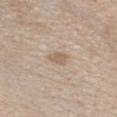{"biopsy_status": "not biopsied; imaged during a skin examination", "lighting": "white-light", "image": {"source": "total-body photography crop", "field_of_view_mm": 15}, "site": "front of the torso", "patient": {"sex": "female", "age_approx": 45}, "lesion_size": {"long_diameter_mm_approx": 2.5}}